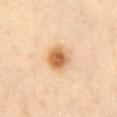The lesion was tiled from a total-body skin photograph and was not biopsied.
A 15 mm crop from a total-body photograph taken for skin-cancer surveillance.
The patient is a female roughly 60 years of age.
The recorded lesion diameter is about 3.5 mm.
The lesion is on the abdomen.